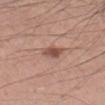Recorded during total-body skin imaging; not selected for excision or biopsy. The subject is a male approximately 45 years of age. Approximately 2.5 mm at its widest. A region of skin cropped from a whole-body photographic capture, roughly 15 mm wide. On the arm. Imaged with white-light lighting. Automated tile analysis of the lesion measured roughly 11 lightness units darker than nearby skin and a lesion-to-skin contrast of about 8 (normalized; higher = more distinct). The software also gave lesion-presence confidence of about 100/100.